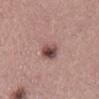notes=total-body-photography surveillance lesion; no biopsy | illumination=white-light | body site=the back | subject=male, approximately 40 years of age | imaging modality=total-body-photography crop, ~15 mm field of view | lesion size=about 5.5 mm.